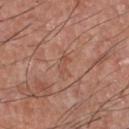workup — imaged on a skin check; not biopsied
location — the upper back
image source — 15 mm crop, total-body photography
patient — male, aged 43 to 47
image-analysis metrics — an average lesion color of about L≈51 a*≈23 b*≈29 (CIELAB); a border-irregularity rating of about 3.5/10, internal color variation of about 0 on a 0–10 scale, and a peripheral color-asymmetry measure near 0
diameter — ≈2.5 mm
illumination — white-light